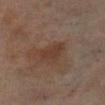Recorded during total-body skin imaging; not selected for excision or biopsy.
The tile uses cross-polarized illumination.
The subject is a female about 70 years old.
Longest diameter approximately 3 mm.
A 15 mm close-up tile from a total-body photography series done for melanoma screening.
The lesion is located on the right lower leg.